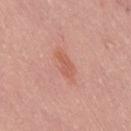Impression:
This lesion was catalogued during total-body skin photography and was not selected for biopsy.
Acquisition and patient details:
Captured under white-light illumination. From the right thigh. A region of skin cropped from a whole-body photographic capture, roughly 15 mm wide. Approximately 3.5 mm at its widest. The patient is a male in their 40s.